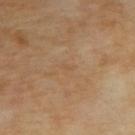Clinical summary: A female subject aged 68 to 72. On the upper back. Cropped from a whole-body photographic skin survey; the tile spans about 15 mm.